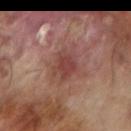The lesion was tiled from a total-body skin photograph and was not biopsied. The lesion's longest dimension is about 4.5 mm. This image is a 15 mm lesion crop taken from a total-body photograph. Located on the right forearm. Automated tile analysis of the lesion measured an area of roughly 9.5 mm², a shape eccentricity near 0.75, and a shape-asymmetry score of about 0.25 (0 = symmetric). It also reported a border-irregularity index near 2.5/10, a color-variation rating of about 3/10, and peripheral color asymmetry of about 1. The analysis additionally found a nevus-likeness score of about 5/100 and a lesion-detection confidence of about 100/100. A male subject about 65 years old. Captured under cross-polarized illumination.